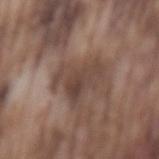Clinical impression: No biopsy was performed on this lesion — it was imaged during a full skin examination and was not determined to be concerning. Context: On the mid back. Approximately 4.5 mm at its widest. A close-up tile cropped from a whole-body skin photograph, about 15 mm across. A male patient in their mid-70s.